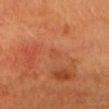{
  "biopsy_status": "not biopsied; imaged during a skin examination",
  "image": {
    "source": "total-body photography crop",
    "field_of_view_mm": 15
  },
  "automated_metrics": {
    "cielab_L": 50,
    "cielab_a": 29,
    "cielab_b": 38,
    "vs_skin_darker_L": 4.0,
    "vs_skin_contrast_norm": 3.5,
    "nevus_likeness_0_100": 0
  },
  "lesion_size": {
    "long_diameter_mm_approx": 1.5
  },
  "lighting": "cross-polarized",
  "patient": {
    "age_approx": 70
  },
  "site": "head or neck"
}